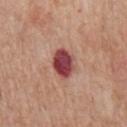Findings:
- follow-up — no biopsy performed (imaged during a skin exam)
- tile lighting — white-light
- subject — male, aged 63–67
- body site — the front of the torso
- automated lesion analysis — a lesion color around L≈45 a*≈30 b*≈22 in CIELAB, about 18 CIELAB-L* units darker than the surrounding skin, and a normalized border contrast of about 13; a lesion-detection confidence of about 100/100
- acquisition — 15 mm crop, total-body photography
- lesion diameter — about 4 mm